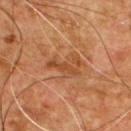Q: Was this lesion biopsied?
A: total-body-photography surveillance lesion; no biopsy
Q: What is the imaging modality?
A: ~15 mm tile from a whole-body skin photo
Q: Automated lesion metrics?
A: a lesion area of about 5.5 mm²; a mean CIELAB color near L≈44 a*≈24 b*≈36, roughly 8 lightness units darker than nearby skin, and a lesion-to-skin contrast of about 6.5 (normalized; higher = more distinct); border irregularity of about 6.5 on a 0–10 scale and a within-lesion color-variation index near 1/10; a detector confidence of about 100 out of 100 that the crop contains a lesion
Q: Lesion location?
A: the chest
Q: What lighting was used for the tile?
A: cross-polarized
Q: What is the lesion's diameter?
A: ~4 mm (longest diameter)
Q: What are the patient's age and sex?
A: male, about 55 years old